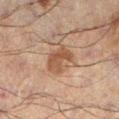notes — total-body-photography surveillance lesion; no biopsy
lesion diameter — ≈4 mm
subject — male, aged around 60
location — the leg
imaging modality — ~15 mm tile from a whole-body skin photo
illumination — cross-polarized illumination